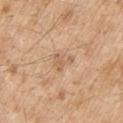A male patient, about 70 years old. Located on the upper back. Captured under white-light illumination. A 15 mm close-up tile from a total-body photography series done for melanoma screening.